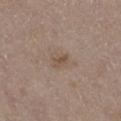A male patient aged approximately 75.
The lesion is located on the right thigh.
About 2.5 mm across.
Imaged with white-light lighting.
A close-up tile cropped from a whole-body skin photograph, about 15 mm across.